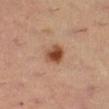automated_metrics:
  area_mm2_approx: 5.5
  eccentricity: 0.75
  shape_asymmetry: 0.2
  nevus_likeness_0_100: 100
lesion_size:
  long_diameter_mm_approx: 3.0
site: right lower leg
image:
  source: total-body photography crop
  field_of_view_mm: 15
lighting: cross-polarized
patient:
  sex: female
  age_approx: 35